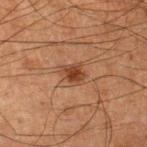This lesion was catalogued during total-body skin photography and was not selected for biopsy. On the right lower leg. A male subject about 65 years old. A 15 mm close-up tile from a total-body photography series done for melanoma screening. Imaged with cross-polarized lighting. Automated tile analysis of the lesion measured a shape eccentricity near 0.6 and a shape-asymmetry score of about 0.25 (0 = symmetric). It also reported a border-irregularity rating of about 2.5/10, a color-variation rating of about 2.5/10, and a peripheral color-asymmetry measure near 1.